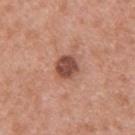biopsy status = total-body-photography surveillance lesion; no biopsy | image-analysis metrics = a lesion color around L≈48 a*≈24 b*≈27 in CIELAB and a normalized border contrast of about 10 | image source = ~15 mm tile from a whole-body skin photo | site = the upper back | subject = male, about 30 years old.